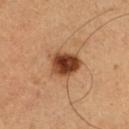{
  "biopsy_status": "not biopsied; imaged during a skin examination",
  "image": {
    "source": "total-body photography crop",
    "field_of_view_mm": 15
  },
  "site": "leg",
  "lighting": "cross-polarized",
  "patient": {
    "sex": "male",
    "age_approx": 50
  },
  "lesion_size": {
    "long_diameter_mm_approx": 4.0
  }
}